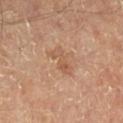This lesion was catalogued during total-body skin photography and was not selected for biopsy.
A patient approximately 55 years of age.
The lesion is located on the right lower leg.
A 15 mm close-up extracted from a 3D total-body photography capture.